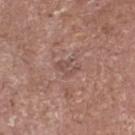Part of a total-body skin-imaging series; this lesion was reviewed on a skin check and was not flagged for biopsy. Automated tile analysis of the lesion measured an outline eccentricity of about 0.7 (0 = round, 1 = elongated) and a symmetry-axis asymmetry near 0.35. It also reported a lesion color around L≈50 a*≈19 b*≈23 in CIELAB and roughly 7 lightness units darker than nearby skin. The software also gave an automated nevus-likeness rating near 0 out of 100 and lesion-presence confidence of about 85/100. The tile uses white-light illumination. Located on the head or neck. This image is a 15 mm lesion crop taken from a total-body photograph. A male patient about 75 years old. The recorded lesion diameter is about 3 mm.